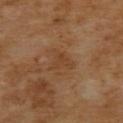Background:
This is a cross-polarized tile. The recorded lesion diameter is about 3 mm. This image is a 15 mm lesion crop taken from a total-body photograph. A female patient, roughly 55 years of age. The lesion is located on the upper back.